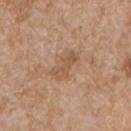Impression:
Part of a total-body skin-imaging series; this lesion was reviewed on a skin check and was not flagged for biopsy.
Clinical summary:
The total-body-photography lesion software estimated an outline eccentricity of about 0.9 (0 = round, 1 = elongated). The software also gave a border-irregularity index near 5/10. Located on the front of the torso. A close-up tile cropped from a whole-body skin photograph, about 15 mm across. The subject is a male approximately 65 years of age. Approximately 3.5 mm at its widest.